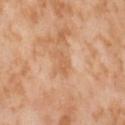Recorded during total-body skin imaging; not selected for excision or biopsy. The patient is a female about 55 years old. The tile uses cross-polarized illumination. A region of skin cropped from a whole-body photographic capture, roughly 15 mm wide. The lesion is located on the left thigh. The lesion's longest dimension is about 3 mm. The lesion-visualizer software estimated an average lesion color of about L≈62 a*≈22 b*≈37 (CIELAB), about 7 CIELAB-L* units darker than the surrounding skin, and a normalized border contrast of about 5. The analysis additionally found a border-irregularity rating of about 4.5/10, a color-variation rating of about 0.5/10, and a peripheral color-asymmetry measure near 0. The software also gave an automated nevus-likeness rating near 0 out of 100.